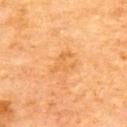Captured during whole-body skin photography for melanoma surveillance; the lesion was not biopsied. Measured at roughly 3.5 mm in maximum diameter. The tile uses cross-polarized illumination. The patient is a male aged around 60. A region of skin cropped from a whole-body photographic capture, roughly 15 mm wide. From the upper back.